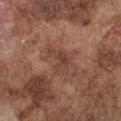Clinical impression:
Part of a total-body skin-imaging series; this lesion was reviewed on a skin check and was not flagged for biopsy.
Context:
Automated image analysis of the tile measured a footprint of about 6 mm², a shape eccentricity near 0.8, and two-axis asymmetry of about 0.5. From the chest. A male subject aged 73 to 77. Measured at roughly 4 mm in maximum diameter. A region of skin cropped from a whole-body photographic capture, roughly 15 mm wide.